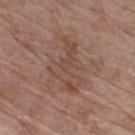This lesion was catalogued during total-body skin photography and was not selected for biopsy.
A 15 mm close-up tile from a total-body photography series done for melanoma screening.
Located on the right thigh.
A female patient in their 70s.